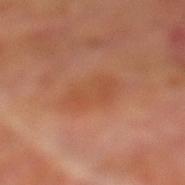Impression:
The lesion was tiled from a total-body skin photograph and was not biopsied.
Background:
A male subject, approximately 70 years of age. A 15 mm crop from a total-body photograph taken for skin-cancer surveillance. From the left lower leg. The total-body-photography lesion software estimated an outline eccentricity of about 0.75 (0 = round, 1 = elongated) and a shape-asymmetry score of about 0.2 (0 = symmetric). The software also gave a normalized lesion–skin contrast near 4.5. The tile uses cross-polarized illumination. Measured at roughly 5 mm in maximum diameter.